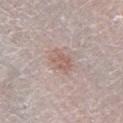Clinical impression: The lesion was photographed on a routine skin check and not biopsied; there is no pathology result. Context: A 15 mm close-up extracted from a 3D total-body photography capture. The lesion is located on the right lower leg. A female patient, in their mid-50s.